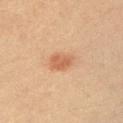anatomic site=the left upper arm | automated lesion analysis=a lesion color around L≈53 a*≈23 b*≈33 in CIELAB, a lesion–skin lightness drop of about 9, and a normalized border contrast of about 7; a within-lesion color-variation index near 1.5/10; a nevus-likeness score of about 95/100 and a detector confidence of about 100 out of 100 that the crop contains a lesion | imaging modality=~15 mm tile from a whole-body skin photo | patient=male, roughly 40 years of age.